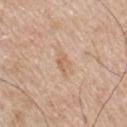Clinical impression: This lesion was catalogued during total-body skin photography and was not selected for biopsy. Acquisition and patient details: From the chest. This is a white-light tile. About 3 mm across. Cropped from a total-body skin-imaging series; the visible field is about 15 mm. A male patient, aged around 80.